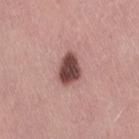The lesion was photographed on a routine skin check and not biopsied; there is no pathology result. The lesion is located on the leg. A region of skin cropped from a whole-body photographic capture, roughly 15 mm wide. A female patient in their 40s. Automated tile analysis of the lesion measured a lesion color around L≈45 a*≈22 b*≈21 in CIELAB, roughly 19 lightness units darker than nearby skin, and a normalized border contrast of about 13. The analysis additionally found border irregularity of about 2.5 on a 0–10 scale and peripheral color asymmetry of about 1. It also reported a nevus-likeness score of about 85/100 and lesion-presence confidence of about 100/100. Imaged with white-light lighting.